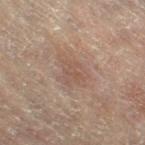Case summary:
– notes: total-body-photography surveillance lesion; no biopsy
– site: the left leg
– patient: female, approximately 80 years of age
– automated lesion analysis: border irregularity of about 4 on a 0–10 scale, a within-lesion color-variation index near 2/10, and a peripheral color-asymmetry measure near 0.5
– size: ≈4.5 mm
– acquisition: ~15 mm crop, total-body skin-cancer survey